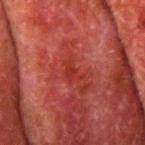No biopsy was performed on this lesion — it was imaged during a full skin examination and was not determined to be concerning. A male patient, aged 78 to 82. On the arm. Longest diameter approximately 2.5 mm. A 15 mm close-up tile from a total-body photography series done for melanoma screening. The lesion-visualizer software estimated a footprint of about 3 mm² and an outline eccentricity of about 0.7 (0 = round, 1 = elongated). The analysis additionally found an average lesion color of about L≈28 a*≈32 b*≈26 (CIELAB), roughly 5 lightness units darker than nearby skin, and a normalized border contrast of about 5.5. The analysis additionally found lesion-presence confidence of about 80/100.